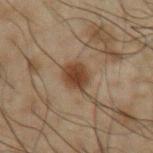<lesion>
<biopsy_status>not biopsied; imaged during a skin examination</biopsy_status>
<lighting>cross-polarized</lighting>
<lesion_size>
  <long_diameter_mm_approx>3.0</long_diameter_mm_approx>
</lesion_size>
<patient>
  <sex>male</sex>
  <age_approx>50</age_approx>
</patient>
<site>arm</site>
<image>
  <source>total-body photography crop</source>
  <field_of_view_mm>15</field_of_view_mm>
</image>
</lesion>